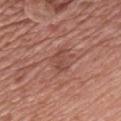This lesion was catalogued during total-body skin photography and was not selected for biopsy. Approximately 3 mm at its widest. An algorithmic analysis of the crop reported a classifier nevus-likeness of about 0/100 and a lesion-detection confidence of about 100/100. Captured under white-light illumination. A male subject in their 50s. The lesion is on the chest. A region of skin cropped from a whole-body photographic capture, roughly 15 mm wide.